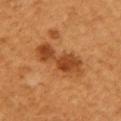A region of skin cropped from a whole-body photographic capture, roughly 15 mm wide.
On the right upper arm.
A female subject aged 53 to 57.